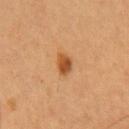biopsy status: catalogued during a skin exam; not biopsied | tile lighting: cross-polarized | imaging modality: ~15 mm tile from a whole-body skin photo | subject: male, roughly 60 years of age | automated metrics: a border-irregularity index near 2.5/10, a within-lesion color-variation index near 4/10, and a peripheral color-asymmetry measure near 1.5 | lesion size: ≈2.5 mm | location: the chest.